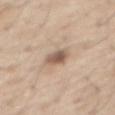Notes:
* workup · imaged on a skin check; not biopsied
* subject · male, in their 70s
* automated metrics · a lesion area of about 5.5 mm², an eccentricity of roughly 0.5, and two-axis asymmetry of about 0.25
* location · the front of the torso
* image · 15 mm crop, total-body photography